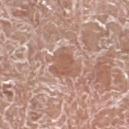| key | value |
|---|---|
| lesion diameter | about 3.5 mm |
| image | ~15 mm crop, total-body skin-cancer survey |
| site | the left lower leg |
| patient | male, aged around 75 |